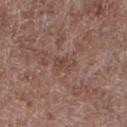<record>
<biopsy_status>not biopsied; imaged during a skin examination</biopsy_status>
<image>
  <source>total-body photography crop</source>
  <field_of_view_mm>15</field_of_view_mm>
</image>
<lighting>white-light</lighting>
<lesion_size>
  <long_diameter_mm_approx>2.5</long_diameter_mm_approx>
</lesion_size>
<site>right lower leg</site>
<patient>
  <sex>male</sex>
  <age_approx>70</age_approx>
</patient>
<automated_metrics>
  <color_variation_0_10>2.5</color_variation_0_10>
  <peripheral_color_asymmetry>1.0</peripheral_color_asymmetry>
  <nevus_likeness_0_100>0</nevus_likeness_0_100>
  <lesion_detection_confidence_0_100>95</lesion_detection_confidence_0_100>
</automated_metrics>
</record>